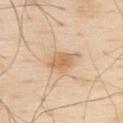About 4 mm across.
A male patient, approximately 80 years of age.
Cropped from a total-body skin-imaging series; the visible field is about 15 mm.
An algorithmic analysis of the crop reported an area of roughly 6.5 mm², an outline eccentricity of about 0.85 (0 = round, 1 = elongated), and a symmetry-axis asymmetry near 0.25.
The lesion is on the upper back.